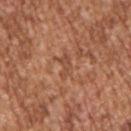Captured during whole-body skin photography for melanoma surveillance; the lesion was not biopsied. A male patient aged approximately 45. The lesion is located on the right upper arm. Approximately 3 mm at its widest. An algorithmic analysis of the crop reported a mean CIELAB color near L≈48 a*≈24 b*≈33, a lesion–skin lightness drop of about 7, and a lesion-to-skin contrast of about 5 (normalized; higher = more distinct). The analysis additionally found an automated nevus-likeness rating near 0 out of 100 and lesion-presence confidence of about 55/100. A roughly 15 mm field-of-view crop from a total-body skin photograph. The tile uses white-light illumination.